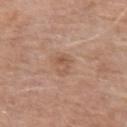No biopsy was performed on this lesion — it was imaged during a full skin examination and was not determined to be concerning. A 15 mm close-up extracted from a 3D total-body photography capture. The lesion is on the arm. The subject is a male in their 70s. Imaged with white-light lighting.